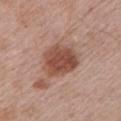This lesion was catalogued during total-body skin photography and was not selected for biopsy.
A male subject, aged around 65.
Approximately 4.5 mm at its widest.
A 15 mm close-up tile from a total-body photography series done for melanoma screening.
On the chest.